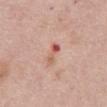Captured during whole-body skin photography for melanoma surveillance; the lesion was not biopsied. About 3 mm across. Cropped from a total-body skin-imaging series; the visible field is about 15 mm. On the abdomen. A female patient in their 60s. The tile uses white-light illumination.